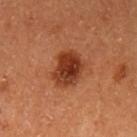Impression: Recorded during total-body skin imaging; not selected for excision or biopsy. Context: Automated tile analysis of the lesion measured about 13 CIELAB-L* units darker than the surrounding skin and a normalized border contrast of about 10.5. And it measured a border-irregularity rating of about 2/10, a color-variation rating of about 5.5/10, and a peripheral color-asymmetry measure near 1.5. Measured at roughly 4.5 mm in maximum diameter. A female patient approximately 50 years of age. The lesion is on the left upper arm. This is a cross-polarized tile. A 15 mm close-up extracted from a 3D total-body photography capture.